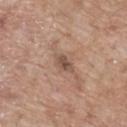Recorded during total-body skin imaging; not selected for excision or biopsy. A lesion tile, about 15 mm wide, cut from a 3D total-body photograph. A male subject, about 70 years old. On the mid back.